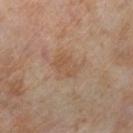Impression: The lesion was photographed on a routine skin check and not biopsied; there is no pathology result. Acquisition and patient details: A female subject, in their mid- to late 50s. On the leg. Imaged with cross-polarized lighting. A 15 mm close-up tile from a total-body photography series done for melanoma screening. The total-body-photography lesion software estimated a lesion area of about 7 mm², an eccentricity of roughly 0.7, and two-axis asymmetry of about 0.2. The software also gave a color-variation rating of about 2.5/10 and peripheral color asymmetry of about 1. About 3.5 mm across.